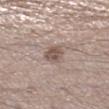image source=total-body-photography crop, ~15 mm field of view
lighting=white-light illumination
location=the right lower leg
image-analysis metrics=an area of roughly 5 mm²
subject=male, roughly 40 years of age
size=~2.5 mm (longest diameter)